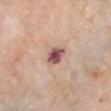This lesion was catalogued during total-body skin photography and was not selected for biopsy. The lesion is on the chest. A female patient approximately 65 years of age. A region of skin cropped from a whole-body photographic capture, roughly 15 mm wide.